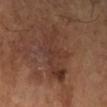Imaged during a routine full-body skin examination; the lesion was not biopsied and no histopathology is available. A region of skin cropped from a whole-body photographic capture, roughly 15 mm wide. Approximately 7.5 mm at its widest. Located on the right lower leg. A male subject approximately 65 years of age.